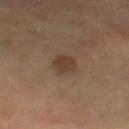Background: The patient is aged around 60. From the right lower leg. The lesion's longest dimension is about 2.5 mm. Captured under cross-polarized illumination. A 15 mm crop from a total-body photograph taken for skin-cancer surveillance. Automated image analysis of the tile measured a footprint of about 5 mm² and a symmetry-axis asymmetry near 0.2.